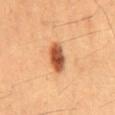Assessment:
Recorded during total-body skin imaging; not selected for excision or biopsy.
Image and clinical context:
The patient is a male roughly 35 years of age. The lesion is on the mid back. A lesion tile, about 15 mm wide, cut from a 3D total-body photograph. The tile uses cross-polarized illumination. About 4 mm across.